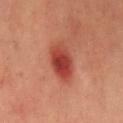Clinical impression: This lesion was catalogued during total-body skin photography and was not selected for biopsy. Image and clinical context: On the chest. A 15 mm crop from a total-body photograph taken for skin-cancer surveillance. Automated image analysis of the tile measured an area of roughly 10 mm², an eccentricity of roughly 0.85, and a shape-asymmetry score of about 0.2 (0 = symmetric). The analysis additionally found a border-irregularity rating of about 2/10 and radial color variation of about 1.5. It also reported a classifier nevus-likeness of about 100/100. About 4.5 mm across. This is a cross-polarized tile. A male patient, in their 40s.